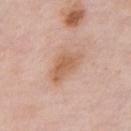Recorded during total-body skin imaging; not selected for excision or biopsy.
A roughly 15 mm field-of-view crop from a total-body skin photograph.
The patient is a male aged around 55.
From the chest.